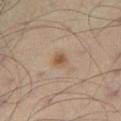This lesion was catalogued during total-body skin photography and was not selected for biopsy. A male patient, approximately 55 years of age. Imaged with cross-polarized lighting. The lesion is located on the right thigh. Cropped from a whole-body photographic skin survey; the tile spans about 15 mm.